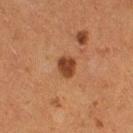biopsy status: no biopsy performed (imaged during a skin exam)
patient: female, about 50 years old
image source: total-body-photography crop, ~15 mm field of view
lighting: cross-polarized
lesion diameter: ~2.5 mm (longest diameter)
location: the right thigh
image-analysis metrics: border irregularity of about 2 on a 0–10 scale, internal color variation of about 2 on a 0–10 scale, and peripheral color asymmetry of about 0.5; an automated nevus-likeness rating near 90 out of 100